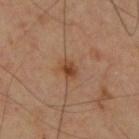biopsy status: imaged on a skin check; not biopsied